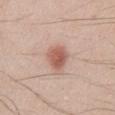| feature | finding |
|---|---|
| workup | no biopsy performed (imaged during a skin exam) |
| subject | male, roughly 25 years of age |
| tile lighting | white-light illumination |
| image source | total-body-photography crop, ~15 mm field of view |
| automated lesion analysis | a border-irregularity index near 1.5/10, internal color variation of about 3.5 on a 0–10 scale, and peripheral color asymmetry of about 1.5 |
| location | the abdomen |